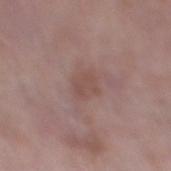This lesion was catalogued during total-body skin photography and was not selected for biopsy.
This is a white-light tile.
The patient is a female in their mid- to late 80s or older.
A 15 mm close-up tile from a total-body photography series done for melanoma screening.
The lesion is located on the right lower leg.
An algorithmic analysis of the crop reported border irregularity of about 2 on a 0–10 scale and internal color variation of about 1 on a 0–10 scale. The analysis additionally found a classifier nevus-likeness of about 0/100.